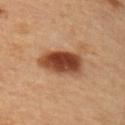workup: total-body-photography surveillance lesion; no biopsy
acquisition: total-body-photography crop, ~15 mm field of view
subject: male, aged 53–57
anatomic site: the right upper arm